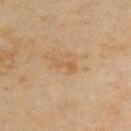Q: Is there a histopathology result?
A: imaged on a skin check; not biopsied
Q: Lesion location?
A: the upper back
Q: What are the patient's age and sex?
A: male, aged approximately 55
Q: How large is the lesion?
A: ~3 mm (longest diameter)
Q: What did automated image analysis measure?
A: a border-irregularity index near 7/10, a color-variation rating of about 0/10, and peripheral color asymmetry of about 0
Q: What is the imaging modality?
A: ~15 mm tile from a whole-body skin photo
Q: How was the tile lit?
A: cross-polarized illumination